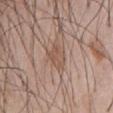Q: Was a biopsy performed?
A: imaged on a skin check; not biopsied
Q: How was the tile lit?
A: white-light
Q: Patient demographics?
A: male, about 60 years old
Q: What is the anatomic site?
A: the chest
Q: What kind of image is this?
A: 15 mm crop, total-body photography